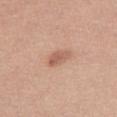biopsy status: imaged on a skin check; not biopsied
size: ≈3 mm
image-analysis metrics: a footprint of about 4 mm², an eccentricity of roughly 0.8, and a shape-asymmetry score of about 0.2 (0 = symmetric); a lesion color around L≈59 a*≈22 b*≈29 in CIELAB, about 10 CIELAB-L* units darker than the surrounding skin, and a normalized border contrast of about 6.5; border irregularity of about 2 on a 0–10 scale, a within-lesion color-variation index near 2.5/10, and a peripheral color-asymmetry measure near 1
site: the leg
lighting: white-light illumination
patient: female, approximately 45 years of age
imaging modality: ~15 mm tile from a whole-body skin photo